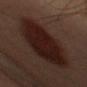Findings:
– follow-up · catalogued during a skin exam; not biopsied
– lighting · cross-polarized
– subject · male, about 50 years old
– lesion size · ~10.5 mm (longest diameter)
– TBP lesion metrics · a footprint of about 37 mm², an outline eccentricity of about 0.9 (0 = round, 1 = elongated), and two-axis asymmetry of about 0.1; about 9 CIELAB-L* units darker than the surrounding skin and a normalized lesion–skin contrast near 12; border irregularity of about 2 on a 0–10 scale and internal color variation of about 3.5 on a 0–10 scale; a nevus-likeness score of about 100/100
– acquisition · 15 mm crop, total-body photography
– location · the left forearm